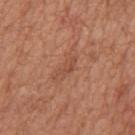biopsy status = catalogued during a skin exam; not biopsied
illumination = white-light
subject = male, about 65 years old
image-analysis metrics = an eccentricity of roughly 0.9 and a symmetry-axis asymmetry near 0.4; a peripheral color-asymmetry measure near 0
diameter = ~3 mm (longest diameter)
image = 15 mm crop, total-body photography
location = the mid back